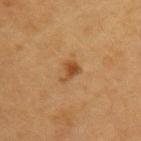Imaged during a routine full-body skin examination; the lesion was not biopsied and no histopathology is available.
A female subject, approximately 40 years of age.
The lesion is on the left upper arm.
A 15 mm close-up tile from a total-body photography series done for melanoma screening.
The tile uses cross-polarized illumination.
Measured at roughly 2.5 mm in maximum diameter.